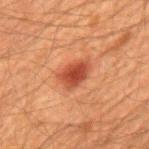follow-up — imaged on a skin check; not biopsied